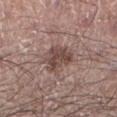follow-up: catalogued during a skin exam; not biopsied | lighting: white-light illumination | subject: male, aged 58 to 62 | size: ≈4 mm | acquisition: ~15 mm tile from a whole-body skin photo | anatomic site: the left lower leg.